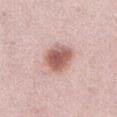  biopsy_status: not biopsied; imaged during a skin examination
  image:
    source: total-body photography crop
    field_of_view_mm: 15
  site: abdomen
  lesion_size:
    long_diameter_mm_approx: 4.0
  patient:
    sex: female
    age_approx: 65